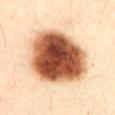biopsy status: imaged on a skin check; not biopsied
diameter: about 7.5 mm
location: the abdomen
patient: female, in their mid-30s
image source: 15 mm crop, total-body photography